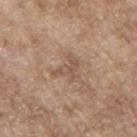Image and clinical context: The patient is a male roughly 65 years of age. A region of skin cropped from a whole-body photographic capture, roughly 15 mm wide. This is a white-light tile. The lesion is located on the right upper arm.